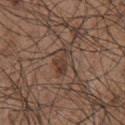Imaged during a routine full-body skin examination; the lesion was not biopsied and no histopathology is available.
This is a white-light tile.
A roughly 15 mm field-of-view crop from a total-body skin photograph.
A male subject, aged approximately 55.
The lesion is located on the chest.